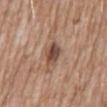The lesion was tiled from a total-body skin photograph and was not biopsied. A lesion tile, about 15 mm wide, cut from a 3D total-body photograph. A male subject, approximately 60 years of age. On the abdomen. An algorithmic analysis of the crop reported a lesion color around L≈47 a*≈19 b*≈27 in CIELAB and roughly 13 lightness units darker than nearby skin. The lesion's longest dimension is about 3.5 mm.